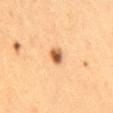Clinical impression:
Imaged during a routine full-body skin examination; the lesion was not biopsied and no histopathology is available.
Clinical summary:
Captured under cross-polarized illumination. Cropped from a total-body skin-imaging series; the visible field is about 15 mm. A female patient, aged around 40. Located on the mid back. The total-body-photography lesion software estimated a lesion area of about 3.5 mm², an eccentricity of roughly 0.8, and a symmetry-axis asymmetry near 0.15. It also reported a border-irregularity index near 1.5/10, internal color variation of about 4.5 on a 0–10 scale, and radial color variation of about 1.5. The software also gave an automated nevus-likeness rating near 100 out of 100 and a detector confidence of about 100 out of 100 that the crop contains a lesion. The lesion's longest dimension is about 2.5 mm.